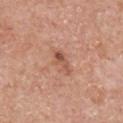biopsy_status: not biopsied; imaged during a skin examination
lighting: white-light
site: chest
image:
  source: total-body photography crop
  field_of_view_mm: 15
patient:
  sex: male
  age_approx: 70
automated_metrics:
  lesion_detection_confidence_0_100: 100
lesion_size:
  long_diameter_mm_approx: 3.5